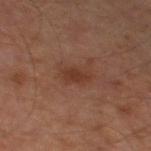Imaged during a routine full-body skin examination; the lesion was not biopsied and no histopathology is available.
A male subject, aged around 55.
On the left thigh.
The tile uses cross-polarized illumination.
Cropped from a whole-body photographic skin survey; the tile spans about 15 mm.
The lesion's longest dimension is about 3 mm.
Automated image analysis of the tile measured an eccentricity of roughly 0.85. It also reported border irregularity of about 2.5 on a 0–10 scale, a color-variation rating of about 1.5/10, and peripheral color asymmetry of about 0.5.